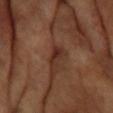Notes:
* follow-up · no biopsy performed (imaged during a skin exam)
* acquisition · ~15 mm tile from a whole-body skin photo
* patient · female, in their mid- to late 70s
* anatomic site · the back